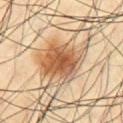{
  "image": {
    "source": "total-body photography crop",
    "field_of_view_mm": 15
  },
  "site": "chest",
  "lesion_size": {
    "long_diameter_mm_approx": 5.5
  },
  "patient": {
    "sex": "male",
    "age_approx": 55
  },
  "lighting": "cross-polarized"
}